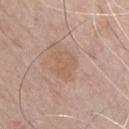Approximately 3.5 mm at its widest. Located on the chest. Imaged with white-light lighting. A roughly 15 mm field-of-view crop from a total-body skin photograph. The patient is a male aged 63 to 67. Automated tile analysis of the lesion measured a color-variation rating of about 2/10 and a peripheral color-asymmetry measure near 0.5. The software also gave a nevus-likeness score of about 0/100 and a detector confidence of about 100 out of 100 that the crop contains a lesion.